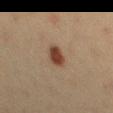The lesion was photographed on a routine skin check and not biopsied; there is no pathology result. A female subject, approximately 40 years of age. Automated image analysis of the tile measured a lesion color around L≈35 a*≈17 b*≈25 in CIELAB and roughly 12 lightness units darker than nearby skin. It also reported lesion-presence confidence of about 100/100. The recorded lesion diameter is about 3 mm. Captured under cross-polarized illumination. A 15 mm close-up tile from a total-body photography series done for melanoma screening. From the left thigh.